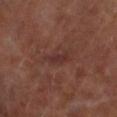Q: Was this lesion biopsied?
A: total-body-photography surveillance lesion; no biopsy
Q: How large is the lesion?
A: ~3 mm (longest diameter)
Q: Who is the patient?
A: male, about 70 years old
Q: How was this image acquired?
A: 15 mm crop, total-body photography
Q: Automated lesion metrics?
A: an area of roughly 3.5 mm², an outline eccentricity of about 0.9 (0 = round, 1 = elongated), and a shape-asymmetry score of about 0.2 (0 = symmetric); an average lesion color of about L≈30 a*≈20 b*≈20 (CIELAB), a lesion–skin lightness drop of about 6, and a normalized lesion–skin contrast near 6; border irregularity of about 2.5 on a 0–10 scale and a peripheral color-asymmetry measure near 0.5
Q: Where on the body is the lesion?
A: the right lower leg